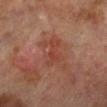The lesion was tiled from a total-body skin photograph and was not biopsied.
A 15 mm close-up tile from a total-body photography series done for melanoma screening.
Measured at roughly 3.5 mm in maximum diameter.
From the left lower leg.
A male patient approximately 70 years of age.